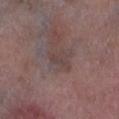Impression:
No biopsy was performed on this lesion — it was imaged during a full skin examination and was not determined to be concerning.
Acquisition and patient details:
From the right lower leg. A male subject, aged approximately 70. A 15 mm crop from a total-body photograph taken for skin-cancer surveillance.